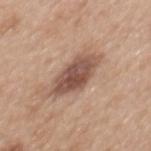This lesion was catalogued during total-body skin photography and was not selected for biopsy.
Longest diameter approximately 6 mm.
The tile uses white-light illumination.
From the mid back.
A roughly 15 mm field-of-view crop from a total-body skin photograph.
A female subject, about 40 years old.
Automated image analysis of the tile measured a lesion color around L≈52 a*≈19 b*≈26 in CIELAB, about 14 CIELAB-L* units darker than the surrounding skin, and a normalized lesion–skin contrast near 9.5. The analysis additionally found a border-irregularity index near 2.5/10, a color-variation rating of about 5/10, and radial color variation of about 1.5. And it measured a nevus-likeness score of about 75/100 and a detector confidence of about 100 out of 100 that the crop contains a lesion.